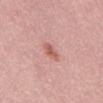A roughly 15 mm field-of-view crop from a total-body skin photograph. From the abdomen. A male subject in their 30s.